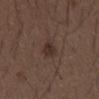No biopsy was performed on this lesion — it was imaged during a full skin examination and was not determined to be concerning.
This is a white-light tile.
A male patient, approximately 50 years of age.
From the abdomen.
The total-body-photography lesion software estimated a mean CIELAB color near L≈30 a*≈14 b*≈20 and a lesion-to-skin contrast of about 8 (normalized; higher = more distinct). The analysis additionally found internal color variation of about 1.5 on a 0–10 scale and radial color variation of about 0.5.
A 15 mm crop from a total-body photograph taken for skin-cancer surveillance.
Measured at roughly 2.5 mm in maximum diameter.